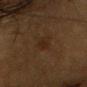<lesion>
  <biopsy_status>not biopsied; imaged during a skin examination</biopsy_status>
  <lesion_size>
    <long_diameter_mm_approx>2.5</long_diameter_mm_approx>
  </lesion_size>
  <automated_metrics>
    <area_mm2_approx>4.0</area_mm2_approx>
    <eccentricity>0.7</eccentricity>
    <shape_asymmetry>0.25</shape_asymmetry>
    <lesion_detection_confidence_0_100>100</lesion_detection_confidence_0_100>
  </automated_metrics>
  <site>chest</site>
  <patient>
    <sex>male</sex>
    <age_approx>85+</age_approx>
  </patient>
  <image>
    <source>total-body photography crop</source>
    <field_of_view_mm>15</field_of_view_mm>
  </image>
  <lighting>cross-polarized</lighting>
</lesion>